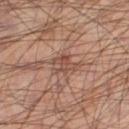Recorded during total-body skin imaging; not selected for excision or biopsy. From the leg. The subject is a male aged 63–67. This image is a 15 mm lesion crop taken from a total-body photograph.